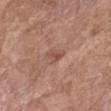  biopsy_status: not biopsied; imaged during a skin examination
  image:
    source: total-body photography crop
    field_of_view_mm: 15
  lesion_size:
    long_diameter_mm_approx: 3.0
  lighting: white-light
  site: left forearm
  patient:
    sex: female
    age_approx: 80
  automated_metrics:
    eccentricity: 0.9
    cielab_L: 51
    cielab_a: 22
    cielab_b: 26
    vs_skin_darker_L: 8.0
    vs_skin_contrast_norm: 5.5
    border_irregularity_0_10: 4.0
    color_variation_0_10: 2.5
    peripheral_color_asymmetry: 1.0